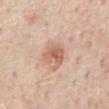The tile uses white-light illumination. The lesion is on the chest. A male subject, about 60 years old. A 15 mm crop from a total-body photograph taken for skin-cancer surveillance.